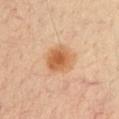This lesion was catalogued during total-body skin photography and was not selected for biopsy. The lesion is located on the right upper arm. A male patient roughly 35 years of age. About 3.5 mm across. Automated image analysis of the tile measured a footprint of about 9.5 mm² and a shape-asymmetry score of about 0.2 (0 = symmetric). It also reported an average lesion color of about L≈55 a*≈21 b*≈36 (CIELAB) and a lesion–skin lightness drop of about 11. A 15 mm close-up extracted from a 3D total-body photography capture. Captured under cross-polarized illumination.